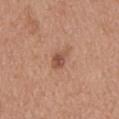Case summary:
– notes · no biopsy performed (imaged during a skin exam)
– patient · female, in their mid-30s
– imaging modality · total-body-photography crop, ~15 mm field of view
– TBP lesion metrics · an automated nevus-likeness rating near 75 out of 100 and a lesion-detection confidence of about 100/100
– anatomic site · the upper back
– lesion diameter · about 2.5 mm